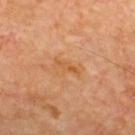workup=no biopsy performed (imaged during a skin exam) | location=the upper back | diameter=about 3.5 mm | image=total-body-photography crop, ~15 mm field of view | lighting=cross-polarized | subject=male, approximately 60 years of age.